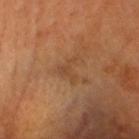Assessment:
Part of a total-body skin-imaging series; this lesion was reviewed on a skin check and was not flagged for biopsy.
Image and clinical context:
The subject is a male aged 58–62. A 15 mm close-up tile from a total-body photography series done for melanoma screening. Imaged with cross-polarized lighting. Measured at roughly 3.5 mm in maximum diameter. The lesion is located on the head or neck.